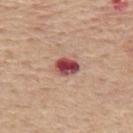This lesion was catalogued during total-body skin photography and was not selected for biopsy.
Cropped from a whole-body photographic skin survey; the tile spans about 15 mm.
A female patient, in their mid-60s.
From the upper back.